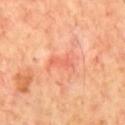| key | value |
|---|---|
| biopsy status | total-body-photography surveillance lesion; no biopsy |
| site | the chest |
| lighting | cross-polarized illumination |
| imaging modality | total-body-photography crop, ~15 mm field of view |
| image-analysis metrics | an average lesion color of about L≈63 a*≈33 b*≈36 (CIELAB), about 7 CIELAB-L* units darker than the surrounding skin, and a normalized border contrast of about 4.5; a border-irregularity rating of about 3/10 and a color-variation rating of about 0.5/10 |
| subject | male, about 70 years old |
| lesion size | ≈3 mm |